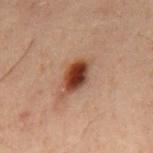Background:
Automated tile analysis of the lesion measured a mean CIELAB color near L≈30 a*≈20 b*≈25 and a normalized lesion–skin contrast near 14. The software also gave a nevus-likeness score of about 100/100 and a detector confidence of about 100 out of 100 that the crop contains a lesion. Captured under cross-polarized illumination. A male patient, aged around 60. The lesion is on the mid back. Longest diameter approximately 3.5 mm. This image is a 15 mm lesion crop taken from a total-body photograph.